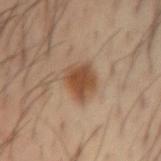This lesion was catalogued during total-body skin photography and was not selected for biopsy. The lesion-visualizer software estimated a lesion area of about 8.5 mm², a shape eccentricity near 0.35, and a symmetry-axis asymmetry near 0.2. The software also gave a classifier nevus-likeness of about 100/100 and a lesion-detection confidence of about 100/100. A male subject about 40 years old. Cropped from a total-body skin-imaging series; the visible field is about 15 mm. Located on the left forearm. About 3.5 mm across.